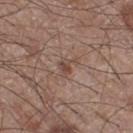Clinical impression:
No biopsy was performed on this lesion — it was imaged during a full skin examination and was not determined to be concerning.
Clinical summary:
A male patient aged approximately 60. The lesion is on the right thigh. Cropped from a whole-body photographic skin survey; the tile spans about 15 mm.